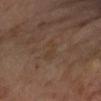notes = no biopsy performed (imaged during a skin exam) | subject = male, aged around 70 | anatomic site = the right forearm | image-analysis metrics = a normalized border contrast of about 4.5; an automated nevus-likeness rating near 0 out of 100 and lesion-presence confidence of about 100/100 | tile lighting = cross-polarized illumination | image = ~15 mm crop, total-body skin-cancer survey.